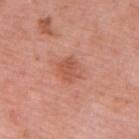This lesion was catalogued during total-body skin photography and was not selected for biopsy.